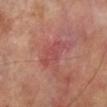A 15 mm crop from a total-body photograph taken for skin-cancer surveillance. Captured under cross-polarized illumination. From the left lower leg. An algorithmic analysis of the crop reported an average lesion color of about L≈47 a*≈29 b*≈24 (CIELAB), roughly 6 lightness units darker than nearby skin, and a normalized lesion–skin contrast near 4.5. A male subject, aged 68–72. The recorded lesion diameter is about 4.5 mm.